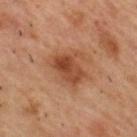follow-up: total-body-photography surveillance lesion; no biopsy | size: ~4.5 mm (longest diameter) | image source: total-body-photography crop, ~15 mm field of view | site: the upper back | subject: male, in their mid-50s | lighting: cross-polarized | automated metrics: a footprint of about 11 mm², an outline eccentricity of about 0.65 (0 = round, 1 = elongated), and a symmetry-axis asymmetry near 0.35; an automated nevus-likeness rating near 65 out of 100 and a lesion-detection confidence of about 100/100.